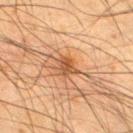biopsy status: imaged on a skin check; not biopsied
lighting: cross-polarized illumination
size: ≈2.5 mm
imaging modality: 15 mm crop, total-body photography
site: the leg
automated metrics: a lesion color around L≈42 a*≈19 b*≈31 in CIELAB and a lesion–skin lightness drop of about 9; border irregularity of about 3 on a 0–10 scale, internal color variation of about 3.5 on a 0–10 scale, and a peripheral color-asymmetry measure near 1.5; an automated nevus-likeness rating near 5 out of 100 and a lesion-detection confidence of about 100/100
patient: male, aged approximately 35